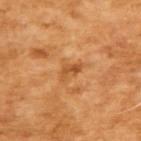Assessment:
Captured during whole-body skin photography for melanoma surveillance; the lesion was not biopsied.
Background:
A male subject, approximately 65 years of age. Cropped from a total-body skin-imaging series; the visible field is about 15 mm.